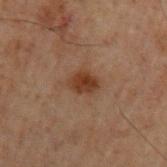{"biopsy_status": "not biopsied; imaged during a skin examination", "site": "arm", "lesion_size": {"long_diameter_mm_approx": 3.0}, "patient": {"sex": "male", "age_approx": 60}, "image": {"source": "total-body photography crop", "field_of_view_mm": 15}, "lighting": "cross-polarized"}